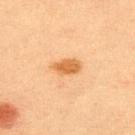workup: no biopsy performed (imaged during a skin exam); lesion size: ≈3 mm; image: ~15 mm crop, total-body skin-cancer survey; subject: male, about 60 years old; automated metrics: a mean CIELAB color near L≈53 a*≈22 b*≈40, roughly 11 lightness units darker than nearby skin, and a normalized lesion–skin contrast near 9; anatomic site: the back; lighting: cross-polarized illumination.